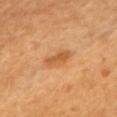Impression: This lesion was catalogued during total-body skin photography and was not selected for biopsy. Background: The lesion's longest dimension is about 3.5 mm. A roughly 15 mm field-of-view crop from a total-body skin photograph. The tile uses cross-polarized illumination. The patient is a female roughly 70 years of age. The lesion is located on the chest. The total-body-photography lesion software estimated a footprint of about 5 mm², an eccentricity of roughly 0.9, and two-axis asymmetry of about 0.25. It also reported a border-irregularity rating of about 2.5/10, internal color variation of about 2 on a 0–10 scale, and radial color variation of about 0.5.